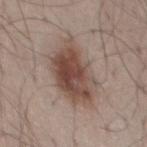No biopsy was performed on this lesion — it was imaged during a full skin examination and was not determined to be concerning.
Imaged with white-light lighting.
The patient is a male approximately 50 years of age.
The recorded lesion diameter is about 7 mm.
Cropped from a whole-body photographic skin survey; the tile spans about 15 mm.
On the mid back.